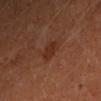The subject is a female in their mid- to late 30s.
From the left forearm.
A 15 mm crop from a total-body photograph taken for skin-cancer surveillance.
Automated tile analysis of the lesion measured a lesion color around L≈29 a*≈22 b*≈27 in CIELAB, a lesion–skin lightness drop of about 6, and a lesion-to-skin contrast of about 6.5 (normalized; higher = more distinct). It also reported an automated nevus-likeness rating near 10 out of 100 and a detector confidence of about 100 out of 100 that the crop contains a lesion.